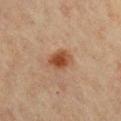{
  "biopsy_status": "not biopsied; imaged during a skin examination"
}